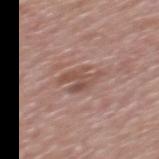Assessment: Captured during whole-body skin photography for melanoma surveillance; the lesion was not biopsied. Context: An algorithmic analysis of the crop reported roughly 9 lightness units darker than nearby skin and a lesion-to-skin contrast of about 6.5 (normalized; higher = more distinct). And it measured a classifier nevus-likeness of about 0/100 and a lesion-detection confidence of about 95/100. A male subject aged 68 to 72. Measured at roughly 4 mm in maximum diameter. The lesion is on the mid back. Cropped from a total-body skin-imaging series; the visible field is about 15 mm. The tile uses white-light illumination.